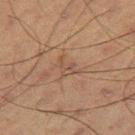Captured during whole-body skin photography for melanoma surveillance; the lesion was not biopsied.
A male patient, about 60 years old.
Imaged with cross-polarized lighting.
An algorithmic analysis of the crop reported a footprint of about 3.5 mm² and two-axis asymmetry of about 0.55.
The recorded lesion diameter is about 3 mm.
Cropped from a whole-body photographic skin survey; the tile spans about 15 mm.
The lesion is located on the right thigh.